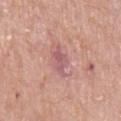Assessment: Captured during whole-body skin photography for melanoma surveillance; the lesion was not biopsied. Clinical summary: Measured at roughly 3.5 mm in maximum diameter. The tile uses white-light illumination. On the left upper arm. A female subject in their mid- to late 60s. A 15 mm close-up tile from a total-body photography series done for melanoma screening.